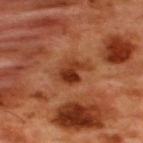notes = total-body-photography surveillance lesion; no biopsy | acquisition = 15 mm crop, total-body photography | patient = male, aged around 50 | automated metrics = a footprint of about 8 mm², a shape eccentricity near 0.7, and two-axis asymmetry of about 0.25; an average lesion color of about L≈38 a*≈28 b*≈36 (CIELAB) and roughly 11 lightness units darker than nearby skin; a classifier nevus-likeness of about 45/100 | site = the back | lighting = cross-polarized illumination.